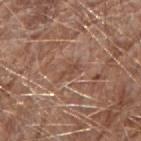No biopsy was performed on this lesion — it was imaged during a full skin examination and was not determined to be concerning.
This image is a 15 mm lesion crop taken from a total-body photograph.
The lesion is located on the right forearm.
A male patient approximately 70 years of age.